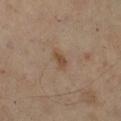workup: catalogued during a skin exam; not biopsied | anatomic site: the right lower leg | image source: total-body-photography crop, ~15 mm field of view | patient: female, aged around 60.